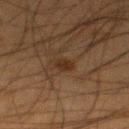Q: Was a biopsy performed?
A: imaged on a skin check; not biopsied
Q: Who is the patient?
A: male, roughly 35 years of age
Q: What is the anatomic site?
A: the left lower leg
Q: What did automated image analysis measure?
A: a nevus-likeness score of about 65/100 and lesion-presence confidence of about 100/100
Q: What is the imaging modality?
A: ~15 mm tile from a whole-body skin photo
Q: Illumination type?
A: cross-polarized illumination